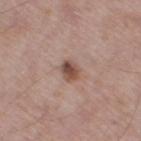Assessment: No biopsy was performed on this lesion — it was imaged during a full skin examination and was not determined to be concerning. Background: The lesion's longest dimension is about 3 mm. Captured under white-light illumination. The subject is a male aged 53 to 57. A lesion tile, about 15 mm wide, cut from a 3D total-body photograph. Located on the right thigh.